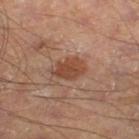Cropped from a total-body skin-imaging series; the visible field is about 15 mm. The recorded lesion diameter is about 4 mm. Automated image analysis of the tile measured an outline eccentricity of about 0.75 (0 = round, 1 = elongated) and a shape-asymmetry score of about 0.25 (0 = symmetric). And it measured a border-irregularity index near 3/10 and peripheral color asymmetry of about 1. The analysis additionally found a nevus-likeness score of about 25/100 and lesion-presence confidence of about 100/100. The patient is a male approximately 55 years of age. From the leg. Captured under cross-polarized illumination.